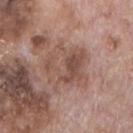The lesion was photographed on a routine skin check and not biopsied; there is no pathology result. A male subject, aged around 70. Measured at roughly 5.5 mm in maximum diameter. Cropped from a total-body skin-imaging series; the visible field is about 15 mm. Imaged with white-light lighting. Located on the chest.